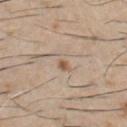<record>
<image>
  <source>total-body photography crop</source>
  <field_of_view_mm>15</field_of_view_mm>
</image>
<patient>
  <sex>male</sex>
  <age_approx>60</age_approx>
</patient>
<site>chest</site>
</record>